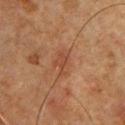Imaged during a routine full-body skin examination; the lesion was not biopsied and no histopathology is available. A male patient, in their 60s. Located on the chest. Cropped from a total-body skin-imaging series; the visible field is about 15 mm.